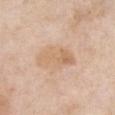Impression: Imaged during a routine full-body skin examination; the lesion was not biopsied and no histopathology is available. Image and clinical context: Cropped from a total-body skin-imaging series; the visible field is about 15 mm. Captured under white-light illumination. The patient is a female approximately 60 years of age. Approximately 5 mm at its widest. From the chest.